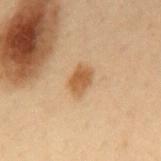Assessment: Imaged during a routine full-body skin examination; the lesion was not biopsied and no histopathology is available. Background: A male subject, aged approximately 55. The tile uses cross-polarized illumination. A region of skin cropped from a whole-body photographic capture, roughly 15 mm wide. The lesion is on the mid back. Longest diameter approximately 3 mm.